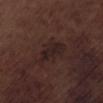biopsy status: imaged on a skin check; not biopsied
image source: 15 mm crop, total-body photography
subject: male, aged approximately 70
location: the left lower leg
TBP lesion metrics: a footprint of about 4.5 mm², an eccentricity of roughly 0.9, and a symmetry-axis asymmetry near 0.2; a mean CIELAB color near L≈20 a*≈14 b*≈15, about 6 CIELAB-L* units darker than the surrounding skin, and a lesion-to-skin contrast of about 8 (normalized; higher = more distinct); a border-irregularity index near 2.5/10, a within-lesion color-variation index near 1.5/10, and peripheral color asymmetry of about 0.5; lesion-presence confidence of about 100/100